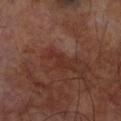{
  "biopsy_status": "not biopsied; imaged during a skin examination",
  "lesion_size": {
    "long_diameter_mm_approx": 3.5
  },
  "patient": {
    "sex": "male",
    "age_approx": 65
  },
  "lighting": "cross-polarized",
  "image": {
    "source": "total-body photography crop",
    "field_of_view_mm": 15
  },
  "site": "left forearm"
}